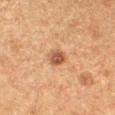Case summary:
• workup: total-body-photography surveillance lesion; no biopsy
• image source: ~15 mm tile from a whole-body skin photo
• lesion diameter: ≈2.5 mm
• lighting: cross-polarized illumination
• site: the left forearm
• subject: female, aged 38 to 42
• automated metrics: a classifier nevus-likeness of about 90/100 and lesion-presence confidence of about 100/100